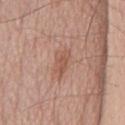<lesion>
  <biopsy_status>not biopsied; imaged during a skin examination</biopsy_status>
  <image>
    <source>total-body photography crop</source>
    <field_of_view_mm>15</field_of_view_mm>
  </image>
  <automated_metrics>
    <area_mm2_approx>5.0</area_mm2_approx>
    <eccentricity>0.85</eccentricity>
    <border_irregularity_0_10>3.5</border_irregularity_0_10>
    <color_variation_0_10>2.5</color_variation_0_10>
    <peripheral_color_asymmetry>1.0</peripheral_color_asymmetry>
    <nevus_likeness_0_100>40</nevus_likeness_0_100>
    <lesion_detection_confidence_0_100>100</lesion_detection_confidence_0_100>
  </automated_metrics>
  <patient>
    <sex>male</sex>
    <age_approx>55</age_approx>
  </patient>
</lesion>